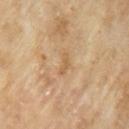Q: Was this lesion biopsied?
A: no biopsy performed (imaged during a skin exam)
Q: What is the imaging modality?
A: total-body-photography crop, ~15 mm field of view
Q: Who is the patient?
A: male, in their 70s
Q: Illumination type?
A: cross-polarized
Q: Automated lesion metrics?
A: an area of roughly 2 mm², an outline eccentricity of about 0.95 (0 = round, 1 = elongated), and a symmetry-axis asymmetry near 0.45; a classifier nevus-likeness of about 0/100 and a lesion-detection confidence of about 100/100
Q: What is the lesion's diameter?
A: ≈3 mm
Q: Where on the body is the lesion?
A: the left upper arm